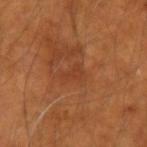{"biopsy_status": "not biopsied; imaged during a skin examination", "site": "left forearm", "lesion_size": {"long_diameter_mm_approx": 2.5}, "patient": {"sex": "male", "age_approx": 50}, "image": {"source": "total-body photography crop", "field_of_view_mm": 15}}